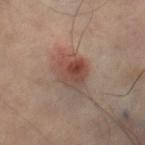Q: Is there a histopathology result?
A: catalogued during a skin exam; not biopsied
Q: Automated lesion metrics?
A: an area of roughly 10 mm², an outline eccentricity of about 0.6 (0 = round, 1 = elongated), and two-axis asymmetry of about 0.15; a mean CIELAB color near L≈47 a*≈21 b*≈26, roughly 10 lightness units darker than nearby skin, and a normalized lesion–skin contrast near 8; a border-irregularity rating of about 1.5/10, a within-lesion color-variation index near 8/10, and a peripheral color-asymmetry measure near 2.5
Q: What kind of image is this?
A: ~15 mm tile from a whole-body skin photo
Q: How was the tile lit?
A: cross-polarized
Q: Lesion size?
A: ≈4 mm
Q: Where on the body is the lesion?
A: the right thigh